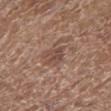lesion size = ≈2.5 mm | patient = female, in their 80s | body site = the left lower leg | image source = 15 mm crop, total-body photography.